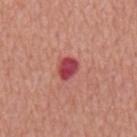Assessment: Recorded during total-body skin imaging; not selected for excision or biopsy. Context: Longest diameter approximately 2.5 mm. This is a white-light tile. This image is a 15 mm lesion crop taken from a total-body photograph. The subject is a male in their mid-60s. The lesion-visualizer software estimated a lesion–skin lightness drop of about 15. The analysis additionally found a nevus-likeness score of about 0/100 and lesion-presence confidence of about 100/100. The lesion is on the mid back.